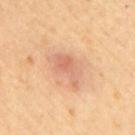Clinical impression: This lesion was catalogued during total-body skin photography and was not selected for biopsy. Image and clinical context: An algorithmic analysis of the crop reported an area of roughly 8 mm², a shape eccentricity near 0.85, and a symmetry-axis asymmetry near 0.35. And it measured about 9 CIELAB-L* units darker than the surrounding skin. The software also gave a border-irregularity index near 4/10, internal color variation of about 4 on a 0–10 scale, and radial color variation of about 1. The tile uses cross-polarized illumination. On the back. A female subject, approximately 65 years of age. Cropped from a whole-body photographic skin survey; the tile spans about 15 mm. Approximately 4.5 mm at its widest.